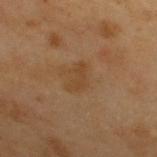Impression: The lesion was tiled from a total-body skin photograph and was not biopsied. Context: From the back. A roughly 15 mm field-of-view crop from a total-body skin photograph. The tile uses cross-polarized illumination. Approximately 3 mm at its widest. The patient is a male approximately 55 years of age.